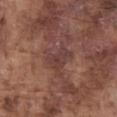Clinical impression:
Recorded during total-body skin imaging; not selected for excision or biopsy.
Clinical summary:
Longest diameter approximately 3.5 mm. This is a white-light tile. The subject is a male roughly 75 years of age. This image is a 15 mm lesion crop taken from a total-body photograph. From the front of the torso. The total-body-photography lesion software estimated a within-lesion color-variation index near 2.5/10 and radial color variation of about 1. The analysis additionally found a nevus-likeness score of about 0/100 and lesion-presence confidence of about 85/100.